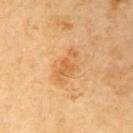Context: A male subject in their 70s. Measured at roughly 4.5 mm in maximum diameter. Captured under cross-polarized illumination. A roughly 15 mm field-of-view crop from a total-body skin photograph. From the right upper arm.